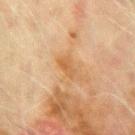Q: Automated lesion metrics?
A: a border-irregularity index near 3/10 and internal color variation of about 1.5 on a 0–10 scale
Q: What are the patient's age and sex?
A: male, aged around 70
Q: How was the tile lit?
A: cross-polarized illumination
Q: Lesion location?
A: the left upper arm
Q: How was this image acquired?
A: 15 mm crop, total-body photography